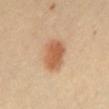Impression: Imaged during a routine full-body skin examination; the lesion was not biopsied and no histopathology is available. Context: A female subject roughly 60 years of age. This image is a 15 mm lesion crop taken from a total-body photograph. Measured at roughly 4.5 mm in maximum diameter. From the chest. The total-body-photography lesion software estimated a lesion area of about 11 mm². The analysis additionally found about 12 CIELAB-L* units darker than the surrounding skin and a normalized border contrast of about 8. The software also gave a border-irregularity index near 1.5/10, internal color variation of about 3.5 on a 0–10 scale, and a peripheral color-asymmetry measure near 1.